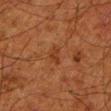{
  "biopsy_status": "not biopsied; imaged during a skin examination",
  "lesion_size": {
    "long_diameter_mm_approx": 2.5
  },
  "automated_metrics": {
    "eccentricity": 0.9,
    "shape_asymmetry": 0.4,
    "cielab_L": 29,
    "cielab_a": 21,
    "cielab_b": 30,
    "vs_skin_darker_L": 6.0,
    "vs_skin_contrast_norm": 6.0,
    "nevus_likeness_0_100": 0,
    "lesion_detection_confidence_0_100": 100
  },
  "lighting": "cross-polarized",
  "patient": {
    "sex": "male",
    "age_approx": 80
  },
  "site": "left lower leg",
  "image": {
    "source": "total-body photography crop",
    "field_of_view_mm": 15
  }
}